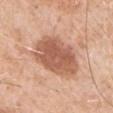Case summary:
- follow-up: catalogued during a skin exam; not biopsied
- illumination: white-light illumination
- TBP lesion metrics: a footprint of about 23 mm², an eccentricity of roughly 0.65, and two-axis asymmetry of about 0.15; roughly 13 lightness units darker than nearby skin and a normalized lesion–skin contrast near 8.5; lesion-presence confidence of about 100/100
- body site: the left upper arm
- image: 15 mm crop, total-body photography
- patient: male, in their 60s
- lesion diameter: ~6 mm (longest diameter)